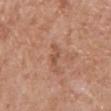Assessment:
Captured during whole-body skin photography for melanoma surveillance; the lesion was not biopsied.
Acquisition and patient details:
On the front of the torso. Approximately 2.5 mm at its widest. The tile uses white-light illumination. A female subject, about 60 years old. Automated image analysis of the tile measured a lesion area of about 2.5 mm² and an outline eccentricity of about 0.85 (0 = round, 1 = elongated). The analysis additionally found an average lesion color of about L≈52 a*≈23 b*≈32 (CIELAB), about 8 CIELAB-L* units darker than the surrounding skin, and a lesion-to-skin contrast of about 6 (normalized; higher = more distinct). The analysis additionally found a detector confidence of about 100 out of 100 that the crop contains a lesion. This image is a 15 mm lesion crop taken from a total-body photograph.